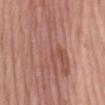notes: total-body-photography surveillance lesion; no biopsy | size: about 13 mm | body site: the mid back | automated lesion analysis: a lesion-detection confidence of about 80/100 | imaging modality: total-body-photography crop, ~15 mm field of view | illumination: white-light illumination | patient: female, in their mid- to late 70s.